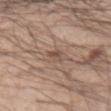{"image": {"source": "total-body photography crop", "field_of_view_mm": 15}, "lesion_size": {"long_diameter_mm_approx": 3.0}, "site": "right forearm", "patient": {"sex": "male", "age_approx": 35}, "lighting": "white-light"}